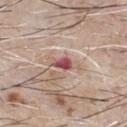Context: On the chest. The patient is a male aged 63–67. The tile uses white-light illumination. This image is a 15 mm lesion crop taken from a total-body photograph. Longest diameter approximately 2.5 mm.